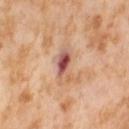Assessment: The lesion was tiled from a total-body skin photograph and was not biopsied. Clinical summary: A 15 mm close-up tile from a total-body photography series done for melanoma screening. Located on the leg. A female subject aged around 55.